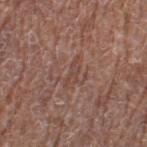No biopsy was performed on this lesion — it was imaged during a full skin examination and was not determined to be concerning.